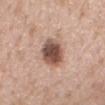Q: Was a biopsy performed?
A: total-body-photography surveillance lesion; no biopsy
Q: How was this image acquired?
A: ~15 mm crop, total-body skin-cancer survey
Q: What is the lesion's diameter?
A: ≈4 mm
Q: Illumination type?
A: white-light
Q: Lesion location?
A: the mid back
Q: Patient demographics?
A: female, aged approximately 50
Q: Automated lesion metrics?
A: a border-irregularity index near 2/10 and a peripheral color-asymmetry measure near 1.5